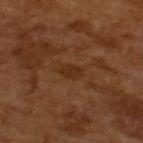<case>
<biopsy_status>not biopsied; imaged during a skin examination</biopsy_status>
<image>
  <source>total-body photography crop</source>
  <field_of_view_mm>15</field_of_view_mm>
</image>
<lighting>cross-polarized</lighting>
<patient>
  <sex>male</sex>
  <age_approx>65</age_approx>
</patient>
<lesion_size>
  <long_diameter_mm_approx>2.5</long_diameter_mm_approx>
</lesion_size>
<automated_metrics>
  <cielab_L>29</cielab_L>
  <cielab_a>20</cielab_a>
  <cielab_b>31</cielab_b>
  <vs_skin_darker_L>6.0</vs_skin_darker_L>
  <vs_skin_contrast_norm>6.5</vs_skin_contrast_norm>
  <border_irregularity_0_10>1.5</border_irregularity_0_10>
  <color_variation_0_10>1.5</color_variation_0_10>
  <peripheral_color_asymmetry>0.5</peripheral_color_asymmetry>
  <nevus_likeness_0_100>0</nevus_likeness_0_100>
</automated_metrics>
</case>